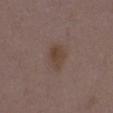Findings:
• follow-up — total-body-photography surveillance lesion; no biopsy